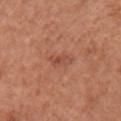Imaged during a routine full-body skin examination; the lesion was not biopsied and no histopathology is available.
Automated image analysis of the tile measured a lesion area of about 3 mm², an eccentricity of roughly 0.9, and a symmetry-axis asymmetry near 0.4. And it measured a lesion–skin lightness drop of about 7 and a normalized border contrast of about 5.5. And it measured a border-irregularity index near 5/10, a within-lesion color-variation index near 0/10, and radial color variation of about 0.
Captured under white-light illumination.
A 15 mm close-up tile from a total-body photography series done for melanoma screening.
A female subject approximately 50 years of age.
Located on the front of the torso.
The lesion's longest dimension is about 3 mm.